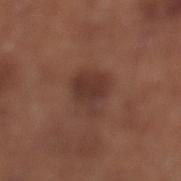This lesion was catalogued during total-body skin photography and was not selected for biopsy. A 15 mm close-up extracted from a 3D total-body photography capture. A male subject aged 68–72. From the leg. The total-body-photography lesion software estimated an average lesion color of about L≈35 a*≈20 b*≈24 (CIELAB) and a lesion–skin lightness drop of about 8. And it measured a border-irregularity index near 3.5/10, internal color variation of about 2.5 on a 0–10 scale, and radial color variation of about 1. The analysis additionally found a classifier nevus-likeness of about 70/100 and lesion-presence confidence of about 100/100. The lesion's longest dimension is about 4 mm. The tile uses white-light illumination.